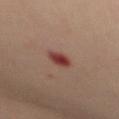Impression: This lesion was catalogued during total-body skin photography and was not selected for biopsy. Acquisition and patient details: On the back. The lesion's longest dimension is about 2.5 mm. Cropped from a whole-body photographic skin survey; the tile spans about 15 mm. A female subject roughly 45 years of age. Imaged with cross-polarized lighting.